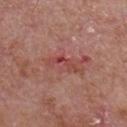<record>
  <biopsy_status>not biopsied; imaged during a skin examination</biopsy_status>
  <lighting>white-light</lighting>
  <image>
    <source>total-body photography crop</source>
    <field_of_view_mm>15</field_of_view_mm>
  </image>
  <patient>
    <sex>male</sex>
    <age_approx>65</age_approx>
  </patient>
  <site>chest</site>
</record>